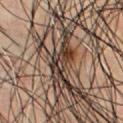| field | value |
|---|---|
| notes | imaged on a skin check; not biopsied |